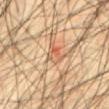This lesion was catalogued during total-body skin photography and was not selected for biopsy. An algorithmic analysis of the crop reported an area of roughly 4 mm², a shape eccentricity near 0.7, and two-axis asymmetry of about 0.25. And it measured border irregularity of about 2.5 on a 0–10 scale, a color-variation rating of about 9/10, and a peripheral color-asymmetry measure near 3.5. And it measured a classifier nevus-likeness of about 0/100 and a detector confidence of about 100 out of 100 that the crop contains a lesion. A 15 mm crop from a total-body photograph taken for skin-cancer surveillance. Captured under cross-polarized illumination. Located on the abdomen. The subject is a male about 45 years old.